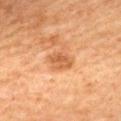| field | value |
|---|---|
| follow-up | catalogued during a skin exam; not biopsied |
| image | total-body-photography crop, ~15 mm field of view |
| lighting | cross-polarized illumination |
| diameter | ~3.5 mm (longest diameter) |
| anatomic site | the back |
| subject | female, aged approximately 60 |